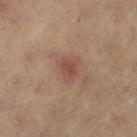No biopsy was performed on this lesion — it was imaged during a full skin examination and was not determined to be concerning. The subject is a female aged 38 to 42. An algorithmic analysis of the crop reported a lesion area of about 2.5 mm² and an outline eccentricity of about 0.55 (0 = round, 1 = elongated). And it measured an average lesion color of about L≈46 a*≈22 b*≈25 (CIELAB), roughly 8 lightness units darker than nearby skin, and a lesion-to-skin contrast of about 6 (normalized; higher = more distinct). A lesion tile, about 15 mm wide, cut from a 3D total-body photograph. Imaged with cross-polarized lighting. On the right thigh. Measured at roughly 2 mm in maximum diameter.